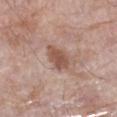Q: Was a biopsy performed?
A: no biopsy performed (imaged during a skin exam)
Q: What is the anatomic site?
A: the leg
Q: Patient demographics?
A: female, aged 68 to 72
Q: What is the lesion's diameter?
A: about 3.5 mm
Q: What is the imaging modality?
A: 15 mm crop, total-body photography
Q: Automated lesion metrics?
A: an area of roughly 6 mm², a shape eccentricity near 0.75, and a shape-asymmetry score of about 0.2 (0 = symmetric); an average lesion color of about L≈52 a*≈21 b*≈27 (CIELAB) and roughly 11 lightness units darker than nearby skin; border irregularity of about 2.5 on a 0–10 scale, a within-lesion color-variation index near 2.5/10, and peripheral color asymmetry of about 1
Q: Illumination type?
A: white-light illumination